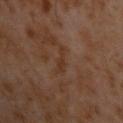The lesion was tiled from a total-body skin photograph and was not biopsied.
The lesion's longest dimension is about 3.5 mm.
A male subject, aged 58 to 62.
A 15 mm close-up tile from a total-body photography series done for melanoma screening.
Located on the chest.